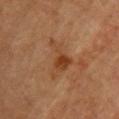biopsy status = catalogued during a skin exam; not biopsied
acquisition = ~15 mm crop, total-body skin-cancer survey
lesion diameter = about 5 mm
site = the upper back
patient = female, aged 58–62
TBP lesion metrics = roughly 7 lightness units darker than nearby skin and a normalized lesion–skin contrast near 7